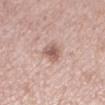workup — total-body-photography surveillance lesion; no biopsy | imaging modality — total-body-photography crop, ~15 mm field of view | illumination — white-light illumination | subject — female, roughly 55 years of age | lesion diameter — ~3 mm (longest diameter) | automated metrics — a shape eccentricity near 0.7 and two-axis asymmetry of about 0.25; an average lesion color of about L≈58 a*≈19 b*≈24 (CIELAB), roughly 12 lightness units darker than nearby skin, and a normalized border contrast of about 8 | body site — the left lower leg.